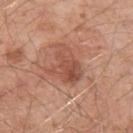Clinical impression:
Captured during whole-body skin photography for melanoma surveillance; the lesion was not biopsied.
Clinical summary:
A male patient, aged approximately 55. Automated tile analysis of the lesion measured a footprint of about 11 mm² and an eccentricity of roughly 0.7. The software also gave a mean CIELAB color near L≈51 a*≈24 b*≈30, roughly 9 lightness units darker than nearby skin, and a lesion-to-skin contrast of about 6.5 (normalized; higher = more distinct). The software also gave a color-variation rating of about 5.5/10 and peripheral color asymmetry of about 2. The software also gave a nevus-likeness score of about 0/100 and a lesion-detection confidence of about 100/100. Located on the left upper arm. About 5 mm across. Captured under white-light illumination. A region of skin cropped from a whole-body photographic capture, roughly 15 mm wide.